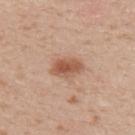notes = total-body-photography surveillance lesion; no biopsy
imaging modality = 15 mm crop, total-body photography
lighting = white-light
subject = female, approximately 30 years of age
automated lesion analysis = an average lesion color of about L≈55 a*≈22 b*≈31 (CIELAB) and roughly 12 lightness units darker than nearby skin; a border-irregularity index near 2/10, internal color variation of about 3.5 on a 0–10 scale, and peripheral color asymmetry of about 1; a nevus-likeness score of about 90/100
site = the upper back
lesion diameter = ~3.5 mm (longest diameter)